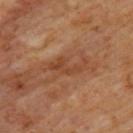The lesion was tiled from a total-body skin photograph and was not biopsied.
A male patient, roughly 60 years of age.
The lesion is on the upper back.
A 15 mm close-up extracted from a 3D total-body photography capture.